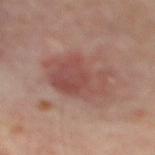follow-up — total-body-photography surveillance lesion; no biopsy | patient — aged 53–57 | location — the mid back | lighting — cross-polarized | imaging modality — ~15 mm tile from a whole-body skin photo.